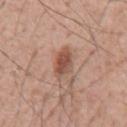Findings:
* workup · catalogued during a skin exam; not biopsied
* patient · male, approximately 55 years of age
* tile lighting · white-light
* anatomic site · the mid back
* automated metrics · roughly 12 lightness units darker than nearby skin and a normalized lesion–skin contrast near 8.5; a border-irregularity index near 1.5/10, a within-lesion color-variation index near 5.5/10, and radial color variation of about 2; a classifier nevus-likeness of about 95/100 and lesion-presence confidence of about 100/100
* image source · 15 mm crop, total-body photography
* lesion diameter · ≈3.5 mm